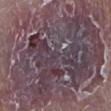Image and clinical context: Imaged with white-light lighting. The lesion's longest dimension is about 15 mm. A 15 mm close-up tile from a total-body photography series done for melanoma screening. A male subject, aged 73–77. The lesion is on the leg.